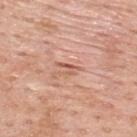A 15 mm crop from a total-body photograph taken for skin-cancer surveillance.
A male subject aged around 60.
The recorded lesion diameter is about 2.5 mm.
Located on the upper back.